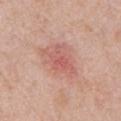Recorded during total-body skin imaging; not selected for excision or biopsy.